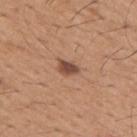| key | value |
|---|---|
| notes | total-body-photography surveillance lesion; no biopsy |
| site | the right upper arm |
| subject | male, aged around 65 |
| imaging modality | ~15 mm tile from a whole-body skin photo |
| image-analysis metrics | an area of roughly 4 mm² and a shape eccentricity near 0.75; a border-irregularity index near 2/10, a color-variation rating of about 3/10, and a peripheral color-asymmetry measure near 1; a nevus-likeness score of about 90/100 |
| lesion diameter | ≈3 mm |
| illumination | white-light illumination |